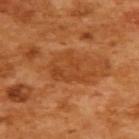{"biopsy_status": "not biopsied; imaged during a skin examination", "lighting": "cross-polarized", "patient": {"sex": "female", "age_approx": 55}, "site": "upper back", "image": {"source": "total-body photography crop", "field_of_view_mm": 15}, "automated_metrics": {"area_mm2_approx": 11.0, "shape_asymmetry": 0.35}}